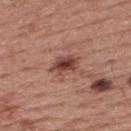| field | value |
|---|---|
| biopsy status | imaged on a skin check; not biopsied |
| subject | male, in their mid- to late 50s |
| anatomic site | the upper back |
| illumination | white-light illumination |
| lesion size | ~3.5 mm (longest diameter) |
| image source | total-body-photography crop, ~15 mm field of view |